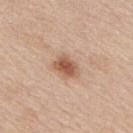{"biopsy_status": "not biopsied; imaged during a skin examination", "image": {"source": "total-body photography crop", "field_of_view_mm": 15}, "site": "upper back", "lesion_size": {"long_diameter_mm_approx": 3.5}, "lighting": "white-light", "patient": {"sex": "male", "age_approx": 60}, "automated_metrics": {"eccentricity": 0.75, "shape_asymmetry": 0.2, "vs_skin_contrast_norm": 8.5, "color_variation_0_10": 3.5, "peripheral_color_asymmetry": 1.0, "lesion_detection_confidence_0_100": 100}}